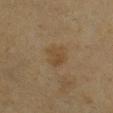Clinical summary: The lesion is located on the right lower leg. Captured under cross-polarized illumination. A close-up tile cropped from a whole-body skin photograph, about 15 mm across. A female subject, aged approximately 55. Longest diameter approximately 3 mm.